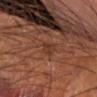Context:
A male patient aged around 70. From the left forearm. Cropped from a total-body skin-imaging series; the visible field is about 15 mm. The tile uses cross-polarized illumination. The lesion's longest dimension is about 2.5 mm.